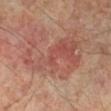lighting: cross-polarized
site: left leg
patient:
  sex: male
  age_approx: 50
lesion_size:
  long_diameter_mm_approx: 9.0
image:
  source: total-body photography crop
  field_of_view_mm: 15